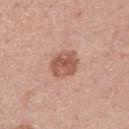Imaged during a routine full-body skin examination; the lesion was not biopsied and no histopathology is available. A male patient, aged 43–47. The tile uses white-light illumination. Measured at roughly 4 mm in maximum diameter. Cropped from a whole-body photographic skin survey; the tile spans about 15 mm. Automated tile analysis of the lesion measured a footprint of about 9 mm² and a shape-asymmetry score of about 0.15 (0 = symmetric). The analysis additionally found internal color variation of about 4.5 on a 0–10 scale and peripheral color asymmetry of about 1.5. The lesion is on the left upper arm.